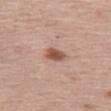Assessment:
Captured during whole-body skin photography for melanoma surveillance; the lesion was not biopsied.
Acquisition and patient details:
From the left thigh. The tile uses white-light illumination. Cropped from a total-body skin-imaging series; the visible field is about 15 mm. A female patient, aged 63 to 67. An algorithmic analysis of the crop reported a lesion area of about 4.5 mm², an eccentricity of roughly 0.65, and a symmetry-axis asymmetry near 0.2. It also reported a lesion color around L≈54 a*≈22 b*≈29 in CIELAB, roughly 13 lightness units darker than nearby skin, and a normalized border contrast of about 9. And it measured a lesion-detection confidence of about 100/100.